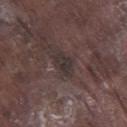Impression:
Recorded during total-body skin imaging; not selected for excision or biopsy.
Clinical summary:
A 15 mm close-up extracted from a 3D total-body photography capture. Measured at roughly 3 mm in maximum diameter. The lesion is located on the right lower leg. A male subject, aged 73 to 77.